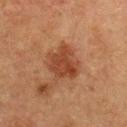<tbp_lesion>
  <biopsy_status>not biopsied; imaged during a skin examination</biopsy_status>
  <lighting>cross-polarized</lighting>
  <lesion_size>
    <long_diameter_mm_approx>4.0</long_diameter_mm_approx>
  </lesion_size>
  <patient>
    <sex>female</sex>
    <age_approx>55</age_approx>
  </patient>
  <image>
    <source>total-body photography crop</source>
    <field_of_view_mm>15</field_of_view_mm>
  </image>
  <site>upper back</site>
</tbp_lesion>